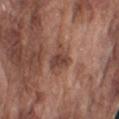follow-up: no biopsy performed (imaged during a skin exam)
patient: male, aged 73–77
imaging modality: ~15 mm crop, total-body skin-cancer survey
automated lesion analysis: an area of roughly 7.5 mm² and two-axis asymmetry of about 0.35; a lesion-to-skin contrast of about 7.5 (normalized; higher = more distinct); a border-irregularity rating of about 3.5/10 and a peripheral color-asymmetry measure near 1.5; a detector confidence of about 100 out of 100 that the crop contains a lesion
tile lighting: white-light
lesion diameter: about 3.5 mm
site: the right upper arm